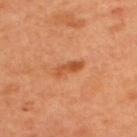Q: Is there a histopathology result?
A: imaged on a skin check; not biopsied